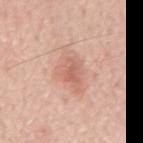| feature | finding |
|---|---|
| workup | catalogued during a skin exam; not biopsied |
| subject | male, approximately 70 years of age |
| diameter | about 5.5 mm |
| image source | total-body-photography crop, ~15 mm field of view |
| location | the mid back |
| automated metrics | about 9 CIELAB-L* units darker than the surrounding skin and a normalized border contrast of about 5.5; a nevus-likeness score of about 5/100 and a lesion-detection confidence of about 100/100 |